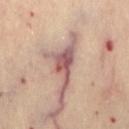Impression:
Captured during whole-body skin photography for melanoma surveillance; the lesion was not biopsied.